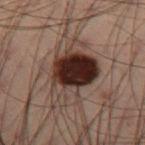Clinical impression: No biopsy was performed on this lesion — it was imaged during a full skin examination and was not determined to be concerning. Context: A male subject, about 55 years old. Imaged with cross-polarized lighting. A 15 mm crop from a total-body photograph taken for skin-cancer surveillance. The total-body-photography lesion software estimated an area of roughly 23 mm² and an outline eccentricity of about 0.75 (0 = round, 1 = elongated). The analysis additionally found a mean CIELAB color near L≈24 a*≈14 b*≈17, a lesion–skin lightness drop of about 15, and a lesion-to-skin contrast of about 15 (normalized; higher = more distinct). And it measured a border-irregularity index near 4/10, a within-lesion color-variation index near 9/10, and peripheral color asymmetry of about 2.5. The analysis additionally found a classifier nevus-likeness of about 85/100 and lesion-presence confidence of about 100/100. Longest diameter approximately 6.5 mm. On the right thigh.